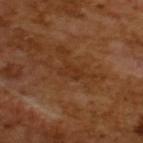Q: Was this lesion biopsied?
A: imaged on a skin check; not biopsied
Q: How was this image acquired?
A: ~15 mm crop, total-body skin-cancer survey
Q: Who is the patient?
A: male, aged 63 to 67
Q: How large is the lesion?
A: ≈2.5 mm